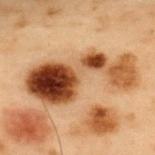Q: Was a biopsy performed?
A: total-body-photography surveillance lesion; no biopsy
Q: What lighting was used for the tile?
A: cross-polarized illumination
Q: What did automated image analysis measure?
A: a footprint of about 35 mm²; an automated nevus-likeness rating near 0 out of 100 and a detector confidence of about 100 out of 100 that the crop contains a lesion
Q: What are the patient's age and sex?
A: male, in their mid-50s
Q: What is the anatomic site?
A: the upper back
Q: What is the imaging modality?
A: ~15 mm tile from a whole-body skin photo